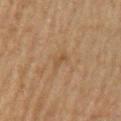Assessment:
This lesion was catalogued during total-body skin photography and was not selected for biopsy.
Context:
The lesion is on the left upper arm. A roughly 15 mm field-of-view crop from a total-body skin photograph. The tile uses cross-polarized illumination. A male patient aged approximately 65.